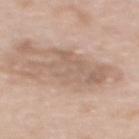Clinical impression:
Imaged during a routine full-body skin examination; the lesion was not biopsied and no histopathology is available.
Background:
A female subject, about 60 years old. A roughly 15 mm field-of-view crop from a total-body skin photograph. The lesion's longest dimension is about 8 mm. Captured under white-light illumination. On the upper back.